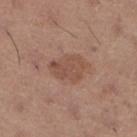<lesion>
<biopsy_status>not biopsied; imaged during a skin examination</biopsy_status>
<automated_metrics>
  <area_mm2_approx>10.0</area_mm2_approx>
  <shape_asymmetry>0.25</shape_asymmetry>
</automated_metrics>
<lighting>white-light</lighting>
<lesion_size>
  <long_diameter_mm_approx>4.0</long_diameter_mm_approx>
</lesion_size>
<site>left lower leg</site>
<image>
  <source>total-body photography crop</source>
  <field_of_view_mm>15</field_of_view_mm>
</image>
<patient>
  <sex>female</sex>
  <age_approx>65</age_approx>
</patient>
</lesion>